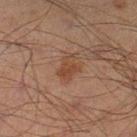No biopsy was performed on this lesion — it was imaged during a full skin examination and was not determined to be concerning.